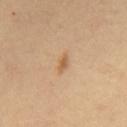<case>
  <biopsy_status>not biopsied; imaged during a skin examination</biopsy_status>
  <lesion_size>
    <long_diameter_mm_approx>2.5</long_diameter_mm_approx>
  </lesion_size>
  <lighting>cross-polarized</lighting>
  <image>
    <source>total-body photography crop</source>
    <field_of_view_mm>15</field_of_view_mm>
  </image>
  <patient>
    <sex>male</sex>
    <age_approx>60</age_approx>
  </patient>
  <site>back</site>
</case>